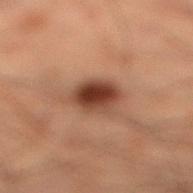No biopsy was performed on this lesion — it was imaged during a full skin examination and was not determined to be concerning. From the leg. A 15 mm crop from a total-body photograph taken for skin-cancer surveillance. Longest diameter approximately 3.5 mm. Captured under cross-polarized illumination. The subject is a male aged around 50.